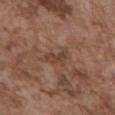Clinical impression: Imaged during a routine full-body skin examination; the lesion was not biopsied and no histopathology is available. Acquisition and patient details: Cropped from a total-body skin-imaging series; the visible field is about 15 mm. Located on the abdomen. A male patient, approximately 75 years of age.